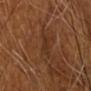{
  "image": {
    "source": "total-body photography crop",
    "field_of_view_mm": 15
  },
  "automated_metrics": {
    "border_irregularity_0_10": 4.5,
    "color_variation_0_10": 1.5,
    "peripheral_color_asymmetry": 0.5
  },
  "patient": {
    "sex": "male",
    "age_approx": 65
  },
  "lighting": "cross-polarized",
  "lesion_size": {
    "long_diameter_mm_approx": 3.5
  },
  "site": "head or neck"
}